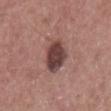workup: imaged on a skin check; not biopsied | size: ~4.5 mm (longest diameter) | subject: male, aged approximately 60 | illumination: white-light | anatomic site: the chest | acquisition: ~15 mm tile from a whole-body skin photo.